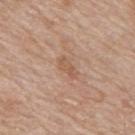The patient is a male aged around 65. Automated tile analysis of the lesion measured a classifier nevus-likeness of about 0/100 and a lesion-detection confidence of about 100/100. The lesion's longest dimension is about 2.5 mm. Cropped from a whole-body photographic skin survey; the tile spans about 15 mm. On the upper back.